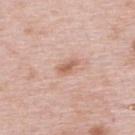Imaged during a routine full-body skin examination; the lesion was not biopsied and no histopathology is available. An algorithmic analysis of the crop reported a footprint of about 3.5 mm². The analysis additionally found a classifier nevus-likeness of about 50/100 and a detector confidence of about 100 out of 100 that the crop contains a lesion. This is a white-light tile. A female patient roughly 65 years of age. A region of skin cropped from a whole-body photographic capture, roughly 15 mm wide. The lesion is on the upper back. The lesion's longest dimension is about 2.5 mm.